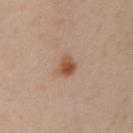Assessment:
Imaged during a routine full-body skin examination; the lesion was not biopsied and no histopathology is available.
Background:
Cropped from a total-body skin-imaging series; the visible field is about 15 mm. From the right upper arm. The subject is a female about 40 years old. The total-body-photography lesion software estimated an area of roughly 4.5 mm², an eccentricity of roughly 0.5, and a symmetry-axis asymmetry near 0.25. The software also gave lesion-presence confidence of about 100/100. Longest diameter approximately 2.5 mm.